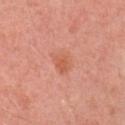Image and clinical context: A male patient aged approximately 45. A 15 mm close-up tile from a total-body photography series done for melanoma screening. On the right upper arm.